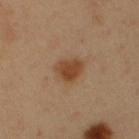{
  "image": {
    "source": "total-body photography crop",
    "field_of_view_mm": 15
  },
  "patient": {
    "sex": "male",
    "age_approx": 50
  },
  "lesion_size": {
    "long_diameter_mm_approx": 3.5
  },
  "lighting": "cross-polarized",
  "site": "abdomen"
}